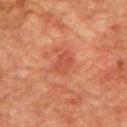| key | value |
|---|---|
| workup | no biopsy performed (imaged during a skin exam) |
| acquisition | ~15 mm crop, total-body skin-cancer survey |
| lesion diameter | about 3 mm |
| subject | male, aged 73 to 77 |
| illumination | cross-polarized illumination |
| image-analysis metrics | an area of roughly 5 mm², an outline eccentricity of about 0.7 (0 = round, 1 = elongated), and two-axis asymmetry of about 0.3; a lesion-detection confidence of about 100/100 |
| body site | the left upper arm |